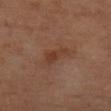workup: catalogued during a skin exam; not biopsied
patient: female, aged 63–67
lighting: cross-polarized illumination
image source: total-body-photography crop, ~15 mm field of view
anatomic site: the left forearm
automated metrics: an area of roughly 5.5 mm², an outline eccentricity of about 0.9 (0 = round, 1 = elongated), and a symmetry-axis asymmetry near 0.4; a mean CIELAB color near L≈38 a*≈21 b*≈28 and a lesion–skin lightness drop of about 7; a border-irregularity index near 4/10, a within-lesion color-variation index near 2/10, and radial color variation of about 0.5
lesion size: about 3.5 mm